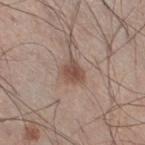follow-up = imaged on a skin check; not biopsied | imaging modality = ~15 mm tile from a whole-body skin photo | diameter = ≈3 mm | subject = male, in their 60s | body site = the right thigh.